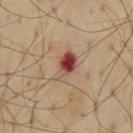  biopsy_status: not biopsied; imaged during a skin examination
  patient:
    sex: male
    age_approx: 55
  site: mid back
  lighting: cross-polarized
  image:
    source: total-body photography crop
    field_of_view_mm: 15